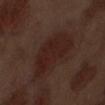notes = no biopsy performed (imaged during a skin exam)
anatomic site = the abdomen
image = total-body-photography crop, ~15 mm field of view
lesion diameter = about 7 mm
subject = male, roughly 70 years of age
image-analysis metrics = an average lesion color of about L≈22 a*≈18 b*≈21 (CIELAB), roughly 6 lightness units darker than nearby skin, and a normalized lesion–skin contrast near 7; a border-irregularity rating of about 3.5/10 and a peripheral color-asymmetry measure near 1; an automated nevus-likeness rating near 5 out of 100 and lesion-presence confidence of about 100/100
illumination = white-light illumination